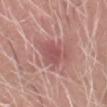This lesion was catalogued during total-body skin photography and was not selected for biopsy.
A lesion tile, about 15 mm wide, cut from a 3D total-body photograph.
A male patient aged 38–42.
Located on the head or neck.
Imaged with white-light lighting.
The lesion-visualizer software estimated an average lesion color of about L≈53 a*≈26 b*≈22 (CIELAB) and a normalized border contrast of about 6.
Approximately 4.5 mm at its widest.